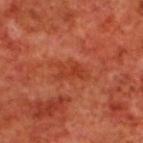Q: Was a biopsy performed?
A: total-body-photography surveillance lesion; no biopsy
Q: How was the tile lit?
A: cross-polarized illumination
Q: Lesion location?
A: the upper back
Q: Patient demographics?
A: male, aged 68 to 72
Q: How large is the lesion?
A: ≈4 mm
Q: Automated lesion metrics?
A: a classifier nevus-likeness of about 0/100 and a lesion-detection confidence of about 100/100
Q: How was this image acquired?
A: total-body-photography crop, ~15 mm field of view